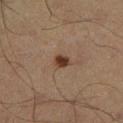Cropped from a whole-body photographic skin survey; the tile spans about 15 mm. A male patient, aged 58 to 62. From the left lower leg.